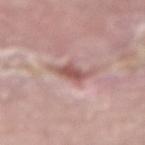Part of a total-body skin-imaging series; this lesion was reviewed on a skin check and was not flagged for biopsy. Cropped from a whole-body photographic skin survey; the tile spans about 15 mm. A male subject, aged 48–52. The lesion is located on the arm. Approximately 3.5 mm at its widest. Captured under white-light illumination. Automated tile analysis of the lesion measured a footprint of about 5.5 mm², a shape eccentricity near 0.7, and two-axis asymmetry of about 0.35. It also reported a lesion–skin lightness drop of about 11. And it measured a border-irregularity index near 4.5/10, internal color variation of about 3 on a 0–10 scale, and peripheral color asymmetry of about 1. The analysis additionally found a nevus-likeness score of about 5/100 and lesion-presence confidence of about 100/100.